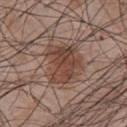Q: Is there a histopathology result?
A: imaged on a skin check; not biopsied
Q: What kind of image is this?
A: ~15 mm crop, total-body skin-cancer survey
Q: What lighting was used for the tile?
A: white-light illumination
Q: What is the lesion's diameter?
A: ~4.5 mm (longest diameter)
Q: Patient demographics?
A: male, approximately 45 years of age
Q: What is the anatomic site?
A: the chest
Q: What did automated image analysis measure?
A: an area of roughly 15 mm², a shape eccentricity near 0.5, and two-axis asymmetry of about 0.4; a lesion color around L≈43 a*≈19 b*≈25 in CIELAB, about 10 CIELAB-L* units darker than the surrounding skin, and a normalized lesion–skin contrast near 8; border irregularity of about 5 on a 0–10 scale and internal color variation of about 4.5 on a 0–10 scale; a classifier nevus-likeness of about 95/100 and a lesion-detection confidence of about 100/100